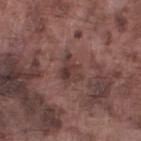The lesion was photographed on a routine skin check and not biopsied; there is no pathology result. Cropped from a whole-body photographic skin survey; the tile spans about 15 mm. The lesion-visualizer software estimated a footprint of about 7.5 mm², a shape eccentricity near 0.5, and two-axis asymmetry of about 0.4. And it measured an average lesion color of about L≈38 a*≈18 b*≈20 (CIELAB), about 8 CIELAB-L* units darker than the surrounding skin, and a lesion-to-skin contrast of about 7 (normalized; higher = more distinct). And it measured internal color variation of about 5.5 on a 0–10 scale and radial color variation of about 2. The analysis additionally found a lesion-detection confidence of about 95/100. This is a white-light tile. The lesion is located on the left thigh. The patient is a male approximately 75 years of age. Measured at roughly 3.5 mm in maximum diameter.